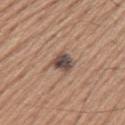• notes: no biopsy performed (imaged during a skin exam)
• patient: male, in their mid-40s
• lesion size: ≈3 mm
• location: the left upper arm
• acquisition: ~15 mm crop, total-body skin-cancer survey